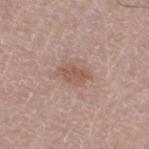This lesion was catalogued during total-body skin photography and was not selected for biopsy. On the right thigh. A male patient, aged approximately 65. A lesion tile, about 15 mm wide, cut from a 3D total-body photograph. Imaged with white-light lighting.